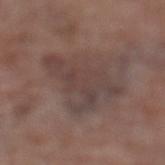notes: no biopsy performed (imaged during a skin exam) | site: the leg | lesion size: ≈6.5 mm | patient: female, aged 78–82 | image: ~15 mm tile from a whole-body skin photo | tile lighting: white-light | automated lesion analysis: a lesion area of about 20 mm², a shape eccentricity near 0.6, and a shape-asymmetry score of about 0.5 (0 = symmetric); an average lesion color of about L≈41 a*≈15 b*≈19 (CIELAB) and a normalized border contrast of about 6; lesion-presence confidence of about 80/100.